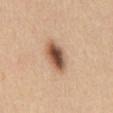<record>
<lesion_size>
  <long_diameter_mm_approx>4.5</long_diameter_mm_approx>
</lesion_size>
<patient>
  <sex>male</sex>
  <age_approx>40</age_approx>
</patient>
<automated_metrics>
  <area_mm2_approx>9.0</area_mm2_approx>
  <shape_asymmetry>0.2</shape_asymmetry>
  <border_irregularity_0_10>2.0</border_irregularity_0_10>
  <peripheral_color_asymmetry>2.5</peripheral_color_asymmetry>
</automated_metrics>
<site>front of the torso</site>
<image>
  <source>total-body photography crop</source>
  <field_of_view_mm>15</field_of_view_mm>
</image>
</record>